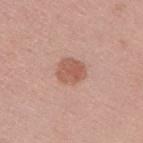Q: Was this lesion biopsied?
A: total-body-photography surveillance lesion; no biopsy
Q: What kind of image is this?
A: ~15 mm tile from a whole-body skin photo
Q: What is the anatomic site?
A: the back
Q: What are the patient's age and sex?
A: male, in their 40s
Q: Illumination type?
A: white-light illumination